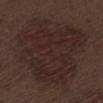Imaged with white-light lighting.
A male patient, aged 68–72.
The lesion is located on the left thigh.
Cropped from a total-body skin-imaging series; the visible field is about 15 mm.